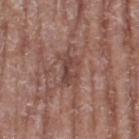| field | value |
|---|---|
| workup | catalogued during a skin exam; not biopsied |
| subject | female, approximately 75 years of age |
| location | the left thigh |
| image | ~15 mm tile from a whole-body skin photo |
| illumination | white-light |
| lesion diameter | about 3.5 mm |
| automated metrics | an area of roughly 6.5 mm² and an eccentricity of roughly 0.8 |